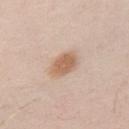Imaged during a routine full-body skin examination; the lesion was not biopsied and no histopathology is available. A 15 mm close-up tile from a total-body photography series done for melanoma screening. The lesion's longest dimension is about 3.5 mm. From the left upper arm. This is a white-light tile. A male patient approximately 30 years of age.